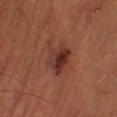Recorded during total-body skin imaging; not selected for excision or biopsy. This is a cross-polarized tile. A lesion tile, about 15 mm wide, cut from a 3D total-body photograph. About 4.5 mm across. From the right lower leg. A male patient aged around 55.